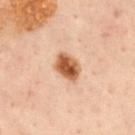Imaged during a routine full-body skin examination; the lesion was not biopsied and no histopathology is available.
A male subject, aged around 50.
Measured at roughly 3.5 mm in maximum diameter.
A close-up tile cropped from a whole-body skin photograph, about 15 mm across.
Captured under cross-polarized illumination.
The lesion is located on the mid back.